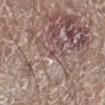The lesion was photographed on a routine skin check and not biopsied; there is no pathology result. Located on the right lower leg. Cropped from a whole-body photographic skin survey; the tile spans about 15 mm. The patient is a male approximately 80 years of age.